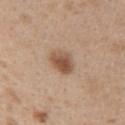Impression:
Imaged during a routine full-body skin examination; the lesion was not biopsied and no histopathology is available.
Acquisition and patient details:
Captured under white-light illumination. From the right upper arm. A female patient roughly 40 years of age. The recorded lesion diameter is about 3 mm. A 15 mm close-up tile from a total-body photography series done for melanoma screening. The lesion-visualizer software estimated a shape eccentricity near 0.65 and two-axis asymmetry of about 0.2. The software also gave a mean CIELAB color near L≈53 a*≈19 b*≈30.